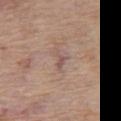workup: catalogued during a skin exam; not biopsied
anatomic site: the left thigh
patient: female, roughly 65 years of age
lesion size: ≈3 mm
imaging modality: ~15 mm crop, total-body skin-cancer survey